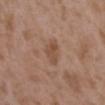Recorded during total-body skin imaging; not selected for excision or biopsy. Located on the upper back. A roughly 15 mm field-of-view crop from a total-body skin photograph. Longest diameter approximately 3.5 mm. The subject is a female aged approximately 35. Captured under white-light illumination.